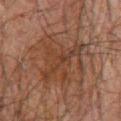Assessment:
Captured during whole-body skin photography for melanoma surveillance; the lesion was not biopsied.
Acquisition and patient details:
The total-body-photography lesion software estimated a lesion color around L≈30 a*≈16 b*≈24 in CIELAB and roughly 5 lightness units darker than nearby skin. The analysis additionally found a border-irregularity index near 8.5/10. And it measured a nevus-likeness score of about 0/100 and lesion-presence confidence of about 90/100. The patient is a male aged around 60. The tile uses cross-polarized illumination. Longest diameter approximately 6.5 mm. On the chest. A 15 mm close-up tile from a total-body photography series done for melanoma screening.